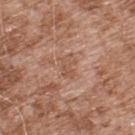Case summary:
• notes: imaged on a skin check; not biopsied
• location: the upper back
• subject: male, approximately 55 years of age
• illumination: white-light illumination
• TBP lesion metrics: a footprint of about 3 mm², an eccentricity of roughly 0.85, and a shape-asymmetry score of about 0.25 (0 = symmetric)
• lesion size: ≈2.5 mm
• image source: ~15 mm tile from a whole-body skin photo